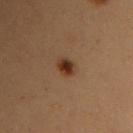No biopsy was performed on this lesion — it was imaged during a full skin examination and was not determined to be concerning. A female patient, aged approximately 40. A lesion tile, about 15 mm wide, cut from a 3D total-body photograph. This is a cross-polarized tile. Located on the arm.